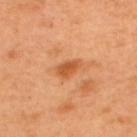{"biopsy_status": "not biopsied; imaged during a skin examination", "lesion_size": {"long_diameter_mm_approx": 3.0}, "image": {"source": "total-body photography crop", "field_of_view_mm": 15}, "site": "upper back", "patient": {"sex": "male", "age_approx": 50}}